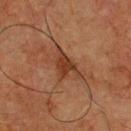Recorded during total-body skin imaging; not selected for excision or biopsy.
A male subject approximately 75 years of age.
The tile uses cross-polarized illumination.
The lesion is on the chest.
The lesion's longest dimension is about 4 mm.
A 15 mm close-up extracted from a 3D total-body photography capture.